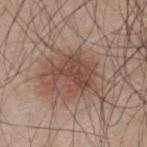This is a white-light tile. Located on the upper back. A male patient aged approximately 45. A 15 mm close-up tile from a total-body photography series done for melanoma screening.